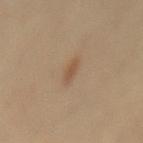workup = imaged on a skin check; not biopsied | tile lighting = cross-polarized | patient = female, aged around 40 | location = the mid back | acquisition = 15 mm crop, total-body photography | lesion size = ≈3 mm.